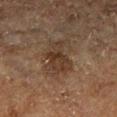Case summary:
- workup — catalogued during a skin exam; not biopsied
- size — ≈5 mm
- location — the right lower leg
- automated metrics — a footprint of about 11 mm², a shape eccentricity near 0.8, and a symmetry-axis asymmetry near 0.5; a lesion color around L≈28 a*≈13 b*≈22 in CIELAB, about 7 CIELAB-L* units darker than the surrounding skin, and a normalized lesion–skin contrast near 7.5; border irregularity of about 5.5 on a 0–10 scale and a peripheral color-asymmetry measure near 1
- image — total-body-photography crop, ~15 mm field of view
- subject — male, aged 73 to 77